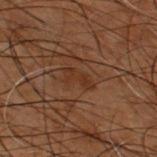follow-up=catalogued during a skin exam; not biopsied | lighting=cross-polarized | automated lesion analysis=a mean CIELAB color near L≈24 a*≈16 b*≈24 and a lesion–skin lightness drop of about 4; a border-irregularity rating of about 5/10 and a color-variation rating of about 1/10; a nevus-likeness score of about 0/100 | body site=the upper back | size=≈3.5 mm | subject=male, aged approximately 50 | image source=~15 mm tile from a whole-body skin photo.